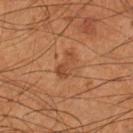No biopsy was performed on this lesion — it was imaged during a full skin examination and was not determined to be concerning.
The lesion-visualizer software estimated an average lesion color of about L≈42 a*≈23 b*≈33 (CIELAB), about 7 CIELAB-L* units darker than the surrounding skin, and a normalized lesion–skin contrast near 6. And it measured a border-irregularity rating of about 5/10, a within-lesion color-variation index near 1/10, and a peripheral color-asymmetry measure near 0.5.
Measured at roughly 3 mm in maximum diameter.
A male subject aged approximately 55.
From the left lower leg.
A roughly 15 mm field-of-view crop from a total-body skin photograph.
This is a cross-polarized tile.